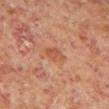Notes:
* notes: total-body-photography surveillance lesion; no biopsy
* site: the left lower leg
* lesion diameter: about 2.5 mm
* acquisition: ~15 mm crop, total-body skin-cancer survey
* subject: male, roughly 60 years of age
* image-analysis metrics: a footprint of about 4 mm² and an outline eccentricity of about 0.7 (0 = round, 1 = elongated); a classifier nevus-likeness of about 0/100 and a detector confidence of about 100 out of 100 that the crop contains a lesion
* tile lighting: cross-polarized